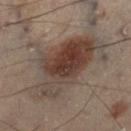workup = catalogued during a skin exam; not biopsied | lighting = cross-polarized illumination | site = the right lower leg | TBP lesion metrics = a lesion area of about 40 mm² and a symmetry-axis asymmetry near 0.3; a nevus-likeness score of about 95/100 and a lesion-detection confidence of about 100/100 | image source = ~15 mm crop, total-body skin-cancer survey | patient = male, aged 53 to 57 | diameter = ~9.5 mm (longest diameter).